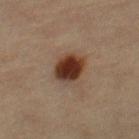No biopsy was performed on this lesion — it was imaged during a full skin examination and was not determined to be concerning. Approximately 4 mm at its widest. A female subject aged 63 to 67. A 15 mm close-up tile from a total-body photography series done for melanoma screening. From the right thigh. An algorithmic analysis of the crop reported a mean CIELAB color near L≈31 a*≈16 b*≈24, about 13 CIELAB-L* units darker than the surrounding skin, and a normalized border contrast of about 12. The software also gave a classifier nevus-likeness of about 100/100.